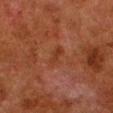biopsy_status: not biopsied; imaged during a skin examination
site: left lower leg
patient:
  sex: male
  age_approx: 80
lighting: cross-polarized
image:
  source: total-body photography crop
  field_of_view_mm: 15
automated_metrics:
  cielab_L: 29
  cielab_a: 21
  cielab_b: 28
  vs_skin_darker_L: 4.0
  vs_skin_contrast_norm: 5.5
lesion_size:
  long_diameter_mm_approx: 3.0